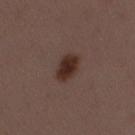Recorded during total-body skin imaging; not selected for excision or biopsy.
On the lower back.
A 15 mm close-up extracted from a 3D total-body photography capture.
A female patient, about 50 years old.